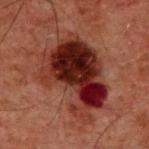Q: Is there a histopathology result?
A: imaged on a skin check; not biopsied
Q: What is the imaging modality?
A: total-body-photography crop, ~15 mm field of view
Q: Who is the patient?
A: male, in their 60s
Q: What did automated image analysis measure?
A: an average lesion color of about L≈22 a*≈23 b*≈21 (CIELAB), about 13 CIELAB-L* units darker than the surrounding skin, and a lesion-to-skin contrast of about 14 (normalized; higher = more distinct); a color-variation rating of about 10/10 and a peripheral color-asymmetry measure near 3.5
Q: How was the tile lit?
A: cross-polarized
Q: What is the anatomic site?
A: the back
Q: What is the lesion's diameter?
A: ≈9 mm